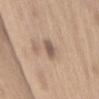  lesion_size:
    long_diameter_mm_approx: 3.0
  patient:
    sex: male
    age_approx: 70
  image:
    source: total-body photography crop
    field_of_view_mm: 15
  automated_metrics:
    area_mm2_approx: 3.5
    shape_asymmetry: 0.25
  site: abdomen
  lighting: white-light